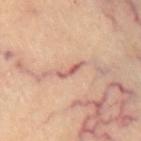<case>
<biopsy_status>not biopsied; imaged during a skin examination</biopsy_status>
<site>chest</site>
<image>
  <source>total-body photography crop</source>
  <field_of_view_mm>15</field_of_view_mm>
</image>
</case>